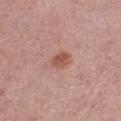Findings:
- biopsy status — imaged on a skin check; not biopsied
- patient — female, aged around 50
- site — the right lower leg
- imaging modality — ~15 mm crop, total-body skin-cancer survey